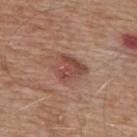notes: no biopsy performed (imaged during a skin exam) | subject: male, aged 63 to 67 | tile lighting: white-light illumination | imaging modality: ~15 mm crop, total-body skin-cancer survey | site: the mid back | size: ≈4 mm | image-analysis metrics: a footprint of about 10 mm²; a border-irregularity index near 3/10, internal color variation of about 4.5 on a 0–10 scale, and a peripheral color-asymmetry measure near 1.5; an automated nevus-likeness rating near 35 out of 100 and a lesion-detection confidence of about 100/100.